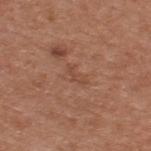{"biopsy_status": "not biopsied; imaged during a skin examination", "site": "upper back", "image": {"source": "total-body photography crop", "field_of_view_mm": 15}, "patient": {"sex": "male", "age_approx": 55}}